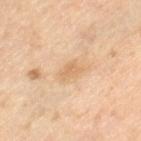On the left thigh.
A male patient approximately 65 years of age.
A region of skin cropped from a whole-body photographic capture, roughly 15 mm wide.
The tile uses cross-polarized illumination.